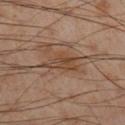Case summary:
- workup · catalogued during a skin exam; not biopsied
- body site · the right lower leg
- lighting · cross-polarized illumination
- TBP lesion metrics · a footprint of about 6 mm², a shape eccentricity near 0.85, and a symmetry-axis asymmetry near 0.4; a border-irregularity index near 4.5/10 and peripheral color asymmetry of about 1; a classifier nevus-likeness of about 0/100
- subject · male, aged 53 to 57
- image source · total-body-photography crop, ~15 mm field of view
- size · ≈4.5 mm